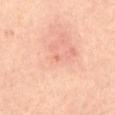workup — no biopsy performed (imaged during a skin exam)
diameter — about 1 mm
automated lesion analysis — a lesion area of about 1 mm², an outline eccentricity of about 0.75 (0 = round, 1 = elongated), and a shape-asymmetry score of about 0.3 (0 = symmetric); an average lesion color of about L≈68 a*≈27 b*≈33 (CIELAB) and a lesion–skin lightness drop of about 5; a border-irregularity index near 2/10 and internal color variation of about 0 on a 0–10 scale; a classifier nevus-likeness of about 0/100 and a detector confidence of about 100 out of 100 that the crop contains a lesion
illumination — cross-polarized illumination
acquisition — 15 mm crop, total-body photography
body site — the front of the torso
patient — female, roughly 45 years of age